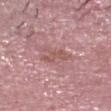Acquisition and patient details:
A male patient, aged approximately 50. An algorithmic analysis of the crop reported border irregularity of about 5.5 on a 0–10 scale, a within-lesion color-variation index near 3.5/10, and peripheral color asymmetry of about 1. Located on the head or neck. A region of skin cropped from a whole-body photographic capture, roughly 15 mm wide.